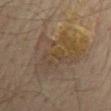This lesion was catalogued during total-body skin photography and was not selected for biopsy.
Approximately 7 mm at its widest.
The patient is a male about 75 years old.
The lesion is on the mid back.
This image is a 15 mm lesion crop taken from a total-body photograph.
The total-body-photography lesion software estimated an average lesion color of about L≈36 a*≈10 b*≈24 (CIELAB) and a normalized border contrast of about 5. The analysis additionally found border irregularity of about 5 on a 0–10 scale, a color-variation rating of about 7.5/10, and radial color variation of about 3.
Imaged with cross-polarized lighting.